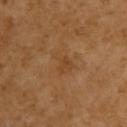Impression: Part of a total-body skin-imaging series; this lesion was reviewed on a skin check and was not flagged for biopsy. Image and clinical context: The lesion is located on the upper back. A roughly 15 mm field-of-view crop from a total-body skin photograph. The patient is a female in their mid-50s. Measured at roughly 3 mm in maximum diameter. Imaged with cross-polarized lighting.